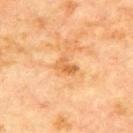* biopsy status: no biopsy performed (imaged during a skin exam)
* image source: ~15 mm crop, total-body skin-cancer survey
* automated lesion analysis: border irregularity of about 4 on a 0–10 scale, a within-lesion color-variation index near 2/10, and peripheral color asymmetry of about 0.5
* anatomic site: the front of the torso
* patient: male, aged approximately 70
* tile lighting: cross-polarized